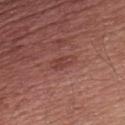follow-up — catalogued during a skin exam; not biopsied | lighting — white-light illumination | image-analysis metrics — an eccentricity of roughly 0.9 and two-axis asymmetry of about 0.25; a lesion color around L≈42 a*≈26 b*≈25 in CIELAB and about 7 CIELAB-L* units darker than the surrounding skin; border irregularity of about 3 on a 0–10 scale, a within-lesion color-variation index near 2/10, and radial color variation of about 0.5 | subject — male, aged approximately 55 | imaging modality — 15 mm crop, total-body photography | anatomic site — the chest.